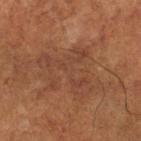Recorded during total-body skin imaging; not selected for excision or biopsy.
Located on the leg.
The patient is a female aged 78–82.
This is a cross-polarized tile.
About 7 mm across.
Automated image analysis of the tile measured an area of roughly 15 mm² and an outline eccentricity of about 0.8 (0 = round, 1 = elongated). The software also gave a border-irregularity rating of about 10/10, a color-variation rating of about 2/10, and peripheral color asymmetry of about 0.5.
Cropped from a total-body skin-imaging series; the visible field is about 15 mm.